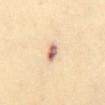Recorded during total-body skin imaging; not selected for excision or biopsy.
The lesion-visualizer software estimated a lesion-detection confidence of about 100/100.
Imaged with cross-polarized lighting.
From the abdomen.
The subject is a female roughly 40 years of age.
A lesion tile, about 15 mm wide, cut from a 3D total-body photograph.